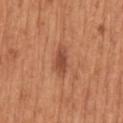A male patient about 65 years old.
A 15 mm close-up extracted from a 3D total-body photography capture.
Captured under white-light illumination.
Located on the mid back.
The total-body-photography lesion software estimated a lesion area of about 5.5 mm², an outline eccentricity of about 0.85 (0 = round, 1 = elongated), and a shape-asymmetry score of about 0.2 (0 = symmetric). It also reported about 10 CIELAB-L* units darker than the surrounding skin. The software also gave a border-irregularity rating of about 2/10, internal color variation of about 3 on a 0–10 scale, and peripheral color asymmetry of about 1. The analysis additionally found a classifier nevus-likeness of about 90/100 and a lesion-detection confidence of about 100/100.